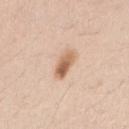This lesion was catalogued during total-body skin photography and was not selected for biopsy. Cropped from a total-body skin-imaging series; the visible field is about 15 mm. The recorded lesion diameter is about 3.5 mm. This is a white-light tile. The patient is a female aged 38 to 42. Automated image analysis of the tile measured an eccentricity of roughly 0.85. It also reported a border-irregularity index near 2/10, a color-variation rating of about 7/10, and peripheral color asymmetry of about 3. The analysis additionally found a classifier nevus-likeness of about 95/100 and lesion-presence confidence of about 100/100. From the right upper arm.